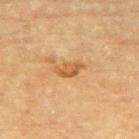biopsy status: no biopsy performed (imaged during a skin exam) | illumination: cross-polarized illumination | image source: ~15 mm tile from a whole-body skin photo | location: the upper back | TBP lesion metrics: a shape eccentricity near 0.85 and a shape-asymmetry score of about 0.35 (0 = symmetric); a border-irregularity index near 3.5/10; a lesion-detection confidence of about 100/100 | patient: female, aged 78 to 82.